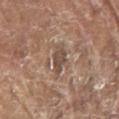Q: Was a biopsy performed?
A: total-body-photography surveillance lesion; no biopsy
Q: Illumination type?
A: white-light illumination
Q: What kind of image is this?
A: ~15 mm crop, total-body skin-cancer survey
Q: Patient demographics?
A: male, about 80 years old
Q: Lesion location?
A: the right upper arm
Q: What is the lesion's diameter?
A: ≈3 mm